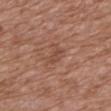<tbp_lesion>
<biopsy_status>not biopsied; imaged during a skin examination</biopsy_status>
<site>mid back</site>
<image>
  <source>total-body photography crop</source>
  <field_of_view_mm>15</field_of_view_mm>
</image>
<lighting>white-light</lighting>
<patient>
  <sex>female</sex>
  <age_approx>75</age_approx>
</patient>
</tbp_lesion>